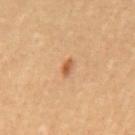| feature | finding |
|---|---|
| follow-up | total-body-photography surveillance lesion; no biopsy |
| location | the mid back |
| acquisition | ~15 mm crop, total-body skin-cancer survey |
| lesion size | ~2 mm (longest diameter) |
| automated lesion analysis | a border-irregularity index near 2.5/10 and a color-variation rating of about 0/10 |
| subject | male, roughly 55 years of age |
| tile lighting | cross-polarized |